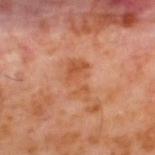Findings:
– notes · imaged on a skin check; not biopsied
– diameter · ~4 mm (longest diameter)
– site · the upper back
– tile lighting · cross-polarized illumination
– automated metrics · an area of roughly 7 mm², an outline eccentricity of about 0.85 (0 = round, 1 = elongated), and a shape-asymmetry score of about 0.5 (0 = symmetric); border irregularity of about 7 on a 0–10 scale, internal color variation of about 2 on a 0–10 scale, and a peripheral color-asymmetry measure near 0.5
– subject · male, aged 58–62
– imaging modality · total-body-photography crop, ~15 mm field of view